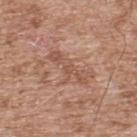Impression:
Recorded during total-body skin imaging; not selected for excision or biopsy.
Acquisition and patient details:
A male patient, in their mid-50s. A roughly 15 mm field-of-view crop from a total-body skin photograph. Located on the upper back.